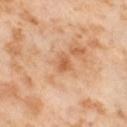Part of a total-body skin-imaging series; this lesion was reviewed on a skin check and was not flagged for biopsy. This is a cross-polarized tile. On the left thigh. A female patient, approximately 55 years of age. A lesion tile, about 15 mm wide, cut from a 3D total-body photograph.